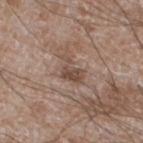No biopsy was performed on this lesion — it was imaged during a full skin examination and was not determined to be concerning. About 3.5 mm across. A 15 mm crop from a total-body photograph taken for skin-cancer surveillance. The lesion is on the left lower leg. The patient is a male in their 60s. This is a white-light tile.